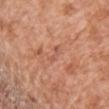Impression:
Captured during whole-body skin photography for melanoma surveillance; the lesion was not biopsied.
Clinical summary:
Automated tile analysis of the lesion measured a lesion area of about 2 mm², an eccentricity of roughly 0.95, and a symmetry-axis asymmetry near 0.6. The software also gave about 7 CIELAB-L* units darker than the surrounding skin and a normalized border contrast of about 5. And it measured border irregularity of about 6.5 on a 0–10 scale, a color-variation rating of about 0/10, and radial color variation of about 0. The analysis additionally found a nevus-likeness score of about 0/100. From the chest. The subject is a male aged approximately 65. A lesion tile, about 15 mm wide, cut from a 3D total-body photograph. Imaged with white-light lighting.